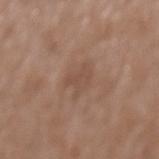Case summary:
– workup — total-body-photography surveillance lesion; no biopsy
– image-analysis metrics — a lesion area of about 5.5 mm², a shape eccentricity near 0.4, and two-axis asymmetry of about 0.4; an average lesion color of about L≈49 a*≈18 b*≈26 (CIELAB) and a lesion-to-skin contrast of about 4.5 (normalized; higher = more distinct)
– image — 15 mm crop, total-body photography
– subject — male, approximately 75 years of age
– size — ≈3 mm
– body site — the mid back